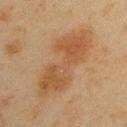Clinical impression:
Imaged during a routine full-body skin examination; the lesion was not biopsied and no histopathology is available.
Acquisition and patient details:
A lesion tile, about 15 mm wide, cut from a 3D total-body photograph. On the arm. The patient is a male in their mid- to late 40s.